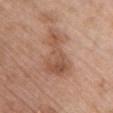biopsy_status: not biopsied; imaged during a skin examination
site: chest
image:
  source: total-body photography crop
  field_of_view_mm: 15
patient:
  sex: female
  age_approx: 60
lesion_size:
  long_diameter_mm_approx: 7.0